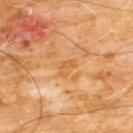Imaged during a routine full-body skin examination; the lesion was not biopsied and no histopathology is available. A region of skin cropped from a whole-body photographic capture, roughly 15 mm wide. From the chest. This is a cross-polarized tile. An algorithmic analysis of the crop reported an outline eccentricity of about 0.85 (0 = round, 1 = elongated) and a shape-asymmetry score of about 0.55 (0 = symmetric). The analysis additionally found a lesion color around L≈58 a*≈24 b*≈45 in CIELAB and a normalized lesion–skin contrast near 5. It also reported a within-lesion color-variation index near 0/10. A male subject, in their 60s.